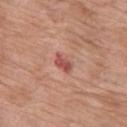Impression: Recorded during total-body skin imaging; not selected for excision or biopsy. Acquisition and patient details: A female subject, about 70 years old. A roughly 15 mm field-of-view crop from a total-body skin photograph. The recorded lesion diameter is about 2.5 mm. This is a white-light tile. From the left thigh.